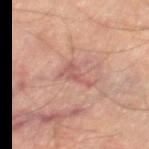Part of a total-body skin-imaging series; this lesion was reviewed on a skin check and was not flagged for biopsy.
The patient is a male in their 70s.
Approximately 4 mm at its widest.
From the right thigh.
This is a cross-polarized tile.
A 15 mm close-up tile from a total-body photography series done for melanoma screening.